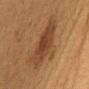Assessment: No biopsy was performed on this lesion — it was imaged during a full skin examination and was not determined to be concerning. Acquisition and patient details: The lesion is on the mid back. A female subject aged around 50. This is a cross-polarized tile. A lesion tile, about 15 mm wide, cut from a 3D total-body photograph. The lesion's longest dimension is about 6.5 mm.